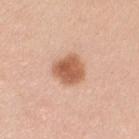| key | value |
|---|---|
| biopsy status | imaged on a skin check; not biopsied |
| anatomic site | the left upper arm |
| imaging modality | 15 mm crop, total-body photography |
| patient | female, in their mid- to late 30s |
| TBP lesion metrics | border irregularity of about 1.5 on a 0–10 scale, a within-lesion color-variation index near 4/10, and a peripheral color-asymmetry measure near 1; a nevus-likeness score of about 100/100 and a lesion-detection confidence of about 100/100 |
| lighting | white-light illumination |